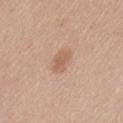Impression: Part of a total-body skin-imaging series; this lesion was reviewed on a skin check and was not flagged for biopsy. Acquisition and patient details: This is a white-light tile. The lesion is on the lower back. The recorded lesion diameter is about 2.5 mm. Automated tile analysis of the lesion measured a lesion–skin lightness drop of about 8 and a normalized lesion–skin contrast near 6. The analysis additionally found a color-variation rating of about 2/10 and radial color variation of about 0.5. The analysis additionally found a classifier nevus-likeness of about 30/100 and a lesion-detection confidence of about 100/100. A close-up tile cropped from a whole-body skin photograph, about 15 mm across. A female patient aged 53–57.